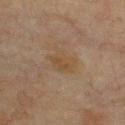{
  "biopsy_status": "not biopsied; imaged during a skin examination",
  "site": "upper back",
  "patient": {
    "sex": "male",
    "age_approx": 65
  },
  "image": {
    "source": "total-body photography crop",
    "field_of_view_mm": 15
  },
  "lighting": "cross-polarized"
}